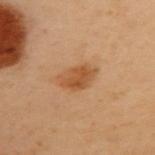The lesion was tiled from a total-body skin photograph and was not biopsied. The lesion is on the back. A female subject aged 58 to 62. Approximately 4 mm at its widest. This is a cross-polarized tile. A roughly 15 mm field-of-view crop from a total-body skin photograph.